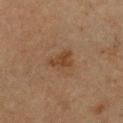The lesion was photographed on a routine skin check and not biopsied; there is no pathology result. A lesion tile, about 15 mm wide, cut from a 3D total-body photograph. The patient is a female approximately 50 years of age. Located on the chest. Imaged with cross-polarized lighting.